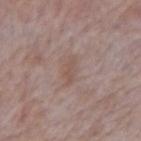This lesion was catalogued during total-body skin photography and was not selected for biopsy.
Measured at roughly 3.5 mm in maximum diameter.
The patient is a male in their mid- to late 60s.
The lesion is located on the right upper arm.
A roughly 15 mm field-of-view crop from a total-body skin photograph.